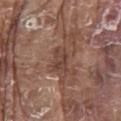Q: Was a biopsy performed?
A: total-body-photography surveillance lesion; no biopsy
Q: Who is the patient?
A: male, aged around 80
Q: How was the tile lit?
A: white-light
Q: How was this image acquired?
A: ~15 mm tile from a whole-body skin photo
Q: Where on the body is the lesion?
A: the mid back